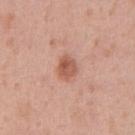Findings:
- biopsy status · imaged on a skin check; not biopsied
- lighting · white-light
- imaging modality · 15 mm crop, total-body photography
- diameter · ≈3 mm
- subject · male, roughly 55 years of age
- body site · the abdomen
- TBP lesion metrics · a lesion color around L≈56 a*≈24 b*≈30 in CIELAB, a lesion–skin lightness drop of about 11, and a normalized border contrast of about 8; an automated nevus-likeness rating near 85 out of 100 and a lesion-detection confidence of about 100/100